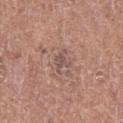A lesion tile, about 15 mm wide, cut from a 3D total-body photograph. A female patient aged 48–52. Located on the leg.